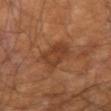Clinical impression:
No biopsy was performed on this lesion — it was imaged during a full skin examination and was not determined to be concerning.
Clinical summary:
A male subject, in their mid- to late 60s. A region of skin cropped from a whole-body photographic capture, roughly 15 mm wide. The lesion is located on the left forearm. This is a cross-polarized tile.